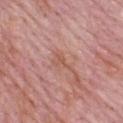Recorded during total-body skin imaging; not selected for excision or biopsy. On the upper back. A male subject, aged around 65. A lesion tile, about 15 mm wide, cut from a 3D total-body photograph. The lesion's longest dimension is about 3 mm.